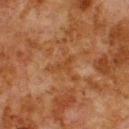Imaged during a routine full-body skin examination; the lesion was not biopsied and no histopathology is available.
On the upper back.
This image is a 15 mm lesion crop taken from a total-body photograph.
A male patient, aged approximately 80.
This is a cross-polarized tile.